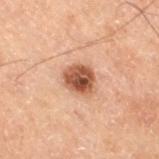biopsy status = no biopsy performed (imaged during a skin exam) | TBP lesion metrics = a lesion area of about 8 mm²; an average lesion color of about L≈40 a*≈20 b*≈26 (CIELAB), a lesion–skin lightness drop of about 14, and a normalized border contrast of about 11; a lesion-detection confidence of about 100/100 | anatomic site = the right thigh | imaging modality = ~15 mm crop, total-body skin-cancer survey | lesion diameter = about 3 mm | subject = male, aged around 70.